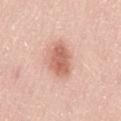Clinical impression: Imaged during a routine full-body skin examination; the lesion was not biopsied and no histopathology is available. Clinical summary: A male patient aged approximately 50. Captured under white-light illumination. Automated image analysis of the tile measured a lesion area of about 8.5 mm² and an eccentricity of roughly 0.75. And it measured an average lesion color of about L≈62 a*≈26 b*≈30 (CIELAB), about 13 CIELAB-L* units darker than the surrounding skin, and a lesion-to-skin contrast of about 8 (normalized; higher = more distinct). And it measured a border-irregularity rating of about 2/10, internal color variation of about 3 on a 0–10 scale, and peripheral color asymmetry of about 1. The lesion is located on the lower back. A 15 mm close-up tile from a total-body photography series done for melanoma screening.